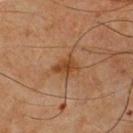The lesion is located on the front of the torso.
An algorithmic analysis of the crop reported a footprint of about 5.5 mm², an outline eccentricity of about 0.65 (0 = round, 1 = elongated), and two-axis asymmetry of about 0.3. The analysis additionally found a mean CIELAB color near L≈38 a*≈19 b*≈32, about 8 CIELAB-L* units darker than the surrounding skin, and a lesion-to-skin contrast of about 7.5 (normalized; higher = more distinct). The analysis additionally found a border-irregularity rating of about 3/10, a color-variation rating of about 2/10, and radial color variation of about 1. It also reported an automated nevus-likeness rating near 15 out of 100 and a lesion-detection confidence of about 100/100.
A male patient, aged 63–67.
A close-up tile cropped from a whole-body skin photograph, about 15 mm across.
Imaged with cross-polarized lighting.
The recorded lesion diameter is about 3 mm.